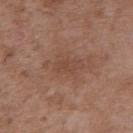notes=catalogued during a skin exam; not biopsied.